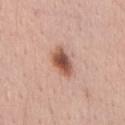| feature | finding |
|---|---|
| follow-up | imaged on a skin check; not biopsied |
| imaging modality | total-body-photography crop, ~15 mm field of view |
| site | the chest |
| size | ≈4 mm |
| image-analysis metrics | a footprint of about 7.5 mm² and a shape eccentricity near 0.8; a lesion color around L≈54 a*≈23 b*≈29 in CIELAB, about 15 CIELAB-L* units darker than the surrounding skin, and a lesion-to-skin contrast of about 10 (normalized; higher = more distinct) |
| patient | male, in their mid- to late 50s |
| tile lighting | white-light illumination |